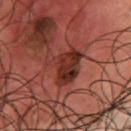The lesion was tiled from a total-body skin photograph and was not biopsied. A lesion tile, about 15 mm wide, cut from a 3D total-body photograph. Measured at roughly 5 mm in maximum diameter. The lesion is on the chest. Captured under cross-polarized illumination. A male patient, about 65 years old.